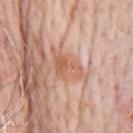follow-up=total-body-photography surveillance lesion; no biopsy
subject=male, roughly 80 years of age
image=~15 mm crop, total-body skin-cancer survey
anatomic site=the chest
lesion diameter=~4.5 mm (longest diameter)